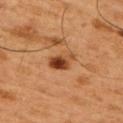Q: Is there a histopathology result?
A: no biopsy performed (imaged during a skin exam)
Q: Where on the body is the lesion?
A: the upper back
Q: Patient demographics?
A: male, about 50 years old
Q: What is the imaging modality?
A: 15 mm crop, total-body photography